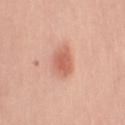No biopsy was performed on this lesion — it was imaged during a full skin examination and was not determined to be concerning.
Cropped from a total-body skin-imaging series; the visible field is about 15 mm.
A female patient roughly 50 years of age.
The recorded lesion diameter is about 3.5 mm.
On the left upper arm.
An algorithmic analysis of the crop reported a lesion area of about 7.5 mm². The software also gave an average lesion color of about L≈62 a*≈27 b*≈31 (CIELAB), a lesion–skin lightness drop of about 11, and a normalized border contrast of about 7. The software also gave a border-irregularity index near 1.5/10, a within-lesion color-variation index near 2.5/10, and a peripheral color-asymmetry measure near 0.5.